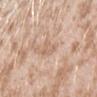No biopsy was performed on this lesion — it was imaged during a full skin examination and was not determined to be concerning. A female subject, in their mid- to late 20s. The lesion's longest dimension is about 2.5 mm. Located on the left forearm. A close-up tile cropped from a whole-body skin photograph, about 15 mm across.